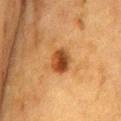biopsy status: catalogued during a skin exam; not biopsied
location: the chest
illumination: cross-polarized illumination
image: ~15 mm tile from a whole-body skin photo
lesion diameter: ≈3.5 mm
image-analysis metrics: a mean CIELAB color near L≈39 a*≈23 b*≈36, about 13 CIELAB-L* units darker than the surrounding skin, and a lesion-to-skin contrast of about 10.5 (normalized; higher = more distinct); an automated nevus-likeness rating near 95 out of 100
subject: female, roughly 55 years of age